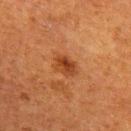This image is a 15 mm lesion crop taken from a total-body photograph. A female patient, about 50 years old. The lesion is located on the right upper arm.